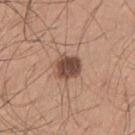biopsy status=catalogued during a skin exam; not biopsied | patient=male, roughly 25 years of age | imaging modality=total-body-photography crop, ~15 mm field of view | body site=the arm.